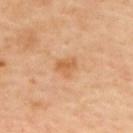follow-up: total-body-photography surveillance lesion; no biopsy
body site: the upper back
subject: male, in their mid- to late 60s
tile lighting: cross-polarized
image: ~15 mm crop, total-body skin-cancer survey
image-analysis metrics: a border-irregularity rating of about 3/10